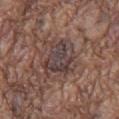biopsy_status: not biopsied; imaged during a skin examination
image:
  source: total-body photography crop
  field_of_view_mm: 15
patient:
  sex: male
  age_approx: 75
lesion_size:
  long_diameter_mm_approx: 5.5
lighting: white-light
site: front of the torso